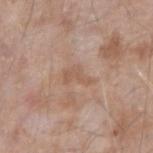Findings:
– notes: catalogued during a skin exam; not biopsied
– diameter: ~3.5 mm (longest diameter)
– illumination: white-light illumination
– subject: male, aged approximately 75
– acquisition: 15 mm crop, total-body photography
– anatomic site: the right forearm